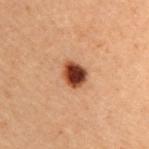Impression:
Recorded during total-body skin imaging; not selected for excision or biopsy.
Context:
The lesion-visualizer software estimated a mean CIELAB color near L≈35 a*≈22 b*≈28, a lesion–skin lightness drop of about 19, and a lesion-to-skin contrast of about 15 (normalized; higher = more distinct). The software also gave a lesion-detection confidence of about 100/100. The tile uses cross-polarized illumination. A close-up tile cropped from a whole-body skin photograph, about 15 mm across. The patient is a female aged around 30. The lesion is located on the upper back. About 3 mm across.